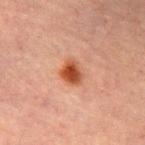Case summary:
- biopsy status · total-body-photography surveillance lesion; no biopsy
- lesion diameter · about 3 mm
- site · the chest
- imaging modality · total-body-photography crop, ~15 mm field of view
- TBP lesion metrics · a border-irregularity index near 1.5/10, a color-variation rating of about 5/10, and radial color variation of about 1.5; a classifier nevus-likeness of about 100/100 and a lesion-detection confidence of about 100/100
- patient · female, roughly 70 years of age
- tile lighting · cross-polarized